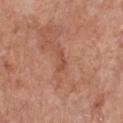<case>
<biopsy_status>not biopsied; imaged during a skin examination</biopsy_status>
<site>chest</site>
<patient>
  <sex>female</sex>
  <age_approx>60</age_approx>
</patient>
<lesion_size>
  <long_diameter_mm_approx>2.5</long_diameter_mm_approx>
</lesion_size>
<image>
  <source>total-body photography crop</source>
  <field_of_view_mm>15</field_of_view_mm>
</image>
</case>